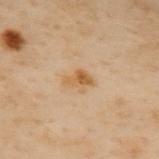<case>
  <biopsy_status>not biopsied; imaged during a skin examination</biopsy_status>
</case>